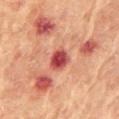<lesion>
<biopsy_status>not biopsied; imaged during a skin examination</biopsy_status>
<image>
  <source>total-body photography crop</source>
  <field_of_view_mm>15</field_of_view_mm>
</image>
<site>front of the torso</site>
<lesion_size>
  <long_diameter_mm_approx>3.0</long_diameter_mm_approx>
</lesion_size>
<patient>
  <sex>female</sex>
  <age_approx>80</age_approx>
</patient>
<lighting>cross-polarized</lighting>
<automated_metrics>
  <area_mm2_approx>6.0</area_mm2_approx>
  <eccentricity>0.65</eccentricity>
  <shape_asymmetry>0.2</shape_asymmetry>
  <cielab_L>46</cielab_L>
  <cielab_a>34</cielab_a>
  <cielab_b>27</cielab_b>
</automated_metrics>
</lesion>